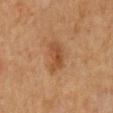acquisition=~15 mm tile from a whole-body skin photo | image-analysis metrics=a border-irregularity rating of about 3.5/10, internal color variation of about 3 on a 0–10 scale, and peripheral color asymmetry of about 1; a classifier nevus-likeness of about 35/100 and a detector confidence of about 100 out of 100 that the crop contains a lesion | body site=the back | lesion diameter=about 4 mm | patient=male, aged around 60 | illumination=cross-polarized.